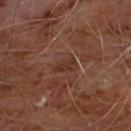Clinical impression:
The lesion was tiled from a total-body skin photograph and was not biopsied.
Context:
Imaged with cross-polarized lighting. Located on the chest. A male patient, in their 60s. Longest diameter approximately 3 mm. A 15 mm crop from a total-body photograph taken for skin-cancer surveillance.